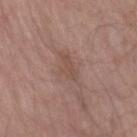– follow-up — no biopsy performed (imaged during a skin exam)
– image source — total-body-photography crop, ~15 mm field of view
– subject — male, roughly 70 years of age
– location — the right forearm